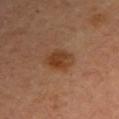Findings:
- biopsy status: no biopsy performed (imaged during a skin exam)
- body site: the right upper arm
- illumination: cross-polarized
- size: ≈3.5 mm
- subject: male, aged approximately 65
- image source: ~15 mm tile from a whole-body skin photo